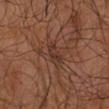Notes:
- TBP lesion metrics · a footprint of about 3.5 mm², an eccentricity of roughly 0.8, and a shape-asymmetry score of about 0.4 (0 = symmetric); a border-irregularity rating of about 4/10, a within-lesion color-variation index near 1.5/10, and radial color variation of about 0.5; a classifier nevus-likeness of about 0/100 and a lesion-detection confidence of about 80/100
- lesion size · ~3 mm (longest diameter)
- subject · male, aged approximately 65
- imaging modality · ~15 mm crop, total-body skin-cancer survey
- site · the right upper arm